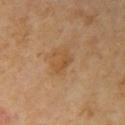{"biopsy_status": "not biopsied; imaged during a skin examination", "patient": {"sex": "female", "age_approx": 60}, "image": {"source": "total-body photography crop", "field_of_view_mm": 15}, "lesion_size": {"long_diameter_mm_approx": 3.0}, "site": "right upper arm", "automated_metrics": {"area_mm2_approx": 4.0, "shape_asymmetry": 0.35, "cielab_L": 50, "cielab_a": 20, "cielab_b": 37, "vs_skin_darker_L": 6.0, "vs_skin_contrast_norm": 5.5, "border_irregularity_0_10": 4.0, "peripheral_color_asymmetry": 0.5}, "lighting": "cross-polarized"}